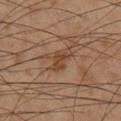follow-up: no biopsy performed (imaged during a skin exam).